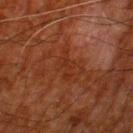Captured during whole-body skin photography for melanoma surveillance; the lesion was not biopsied. The total-body-photography lesion software estimated an area of roughly 4.5 mm² and two-axis asymmetry of about 0.45. And it measured a mean CIELAB color near L≈24 a*≈22 b*≈27, about 4 CIELAB-L* units darker than the surrounding skin, and a lesion-to-skin contrast of about 5 (normalized; higher = more distinct). The software also gave border irregularity of about 6 on a 0–10 scale and peripheral color asymmetry of about 0.5. It also reported an automated nevus-likeness rating near 0 out of 100. Imaged with cross-polarized lighting. From the left thigh. Measured at roughly 3.5 mm in maximum diameter. A male subject, approximately 80 years of age. Cropped from a total-body skin-imaging series; the visible field is about 15 mm.